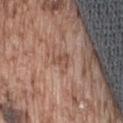Acquisition and patient details: A male patient aged approximately 75. The tile uses white-light illumination. A 15 mm close-up tile from a total-body photography series done for melanoma screening. The lesion is on the lower back.